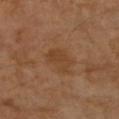Clinical impression: Recorded during total-body skin imaging; not selected for excision or biopsy. Clinical summary: About 3.5 mm across. A female patient roughly 70 years of age. The lesion is on the left forearm. A 15 mm close-up extracted from a 3D total-body photography capture.